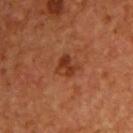patient — male, about 55 years old
lesion size — ~2.5 mm (longest diameter)
image source — 15 mm crop, total-body photography
tile lighting — cross-polarized
anatomic site — the front of the torso